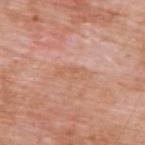Captured during whole-body skin photography for melanoma surveillance; the lesion was not biopsied.
The patient is a male aged 58–62.
The lesion is on the upper back.
Captured under white-light illumination.
Measured at roughly 3.5 mm in maximum diameter.
The total-body-photography lesion software estimated a lesion area of about 3.5 mm², an outline eccentricity of about 0.95 (0 = round, 1 = elongated), and two-axis asymmetry of about 0.35. The software also gave a border-irregularity index near 5/10 and a color-variation rating of about 0/10.
A lesion tile, about 15 mm wide, cut from a 3D total-body photograph.